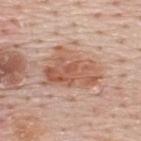Notes:
– workup — catalogued during a skin exam; not biopsied
– subject — male, aged 48–52
– anatomic site — the upper back
– acquisition — total-body-photography crop, ~15 mm field of view